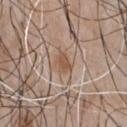A roughly 15 mm field-of-view crop from a total-body skin photograph.
Captured under white-light illumination.
On the chest.
The lesion-visualizer software estimated a lesion area of about 4.5 mm², an eccentricity of roughly 0.65, and a shape-asymmetry score of about 0.3 (0 = symmetric). The software also gave a border-irregularity index near 2.5/10, a color-variation rating of about 3/10, and radial color variation of about 1.
The recorded lesion diameter is about 2.5 mm.
The patient is a male about 50 years old.